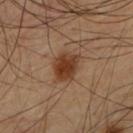Part of a total-body skin-imaging series; this lesion was reviewed on a skin check and was not flagged for biopsy.